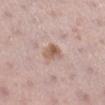The lesion was tiled from a total-body skin photograph and was not biopsied. On the right lower leg. The lesion's longest dimension is about 3 mm. A female subject aged approximately 35. This image is a 15 mm lesion crop taken from a total-body photograph. The tile uses white-light illumination.